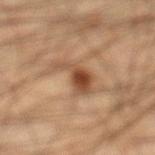Q: What lighting was used for the tile?
A: cross-polarized illumination
Q: What is the imaging modality?
A: ~15 mm tile from a whole-body skin photo
Q: How large is the lesion?
A: ~5 mm (longest diameter)
Q: Lesion location?
A: the left lower leg
Q: Patient demographics?
A: male, in their mid- to late 40s